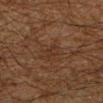This lesion was catalogued during total-body skin photography and was not selected for biopsy. A male patient, in their 60s. Imaged with cross-polarized lighting. From the left lower leg. A close-up tile cropped from a whole-body skin photograph, about 15 mm across. Approximately 2.5 mm at its widest.